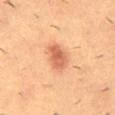Q: Is there a histopathology result?
A: catalogued during a skin exam; not biopsied
Q: How was this image acquired?
A: ~15 mm crop, total-body skin-cancer survey
Q: Where on the body is the lesion?
A: the mid back
Q: What are the patient's age and sex?
A: male, approximately 55 years of age